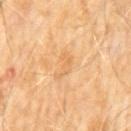Q: Was a biopsy performed?
A: no biopsy performed (imaged during a skin exam)
Q: Patient demographics?
A: male, about 60 years old
Q: What kind of image is this?
A: ~15 mm crop, total-body skin-cancer survey
Q: What is the anatomic site?
A: the abdomen